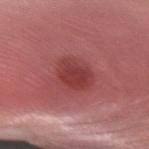notes: no biopsy performed (imaged during a skin exam)
body site: the right forearm
image: ~15 mm crop, total-body skin-cancer survey
automated metrics: an eccentricity of roughly 0.6 and a shape-asymmetry score of about 0.15 (0 = symmetric); a mean CIELAB color near L≈41 a*≈32 b*≈24, a lesion–skin lightness drop of about 9, and a lesion-to-skin contrast of about 7.5 (normalized; higher = more distinct)
subject: male, roughly 45 years of age
tile lighting: white-light illumination